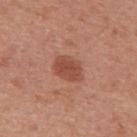Assessment:
This lesion was catalogued during total-body skin photography and was not selected for biopsy.
Background:
Located on the upper back. A female subject approximately 40 years of age. The total-body-photography lesion software estimated an average lesion color of about L≈49 a*≈26 b*≈30 (CIELAB) and about 10 CIELAB-L* units darker than the surrounding skin. The software also gave a classifier nevus-likeness of about 90/100 and a lesion-detection confidence of about 100/100. Captured under white-light illumination. A lesion tile, about 15 mm wide, cut from a 3D total-body photograph. Approximately 3.5 mm at its widest.